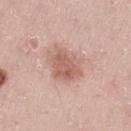workup: catalogued during a skin exam; not biopsied
automated metrics: an average lesion color of about L≈60 a*≈22 b*≈27 (CIELAB) and about 11 CIELAB-L* units darker than the surrounding skin; border irregularity of about 2.5 on a 0–10 scale, a within-lesion color-variation index near 3.5/10, and a peripheral color-asymmetry measure near 1
site: the left thigh
subject: female, about 40 years old
imaging modality: ~15 mm crop, total-body skin-cancer survey
lesion diameter: ≈4 mm
lighting: white-light illumination